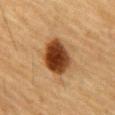Findings:
* follow-up — total-body-photography surveillance lesion; no biopsy
* lesion size — ≈5 mm
* illumination — cross-polarized
* image — ~15 mm tile from a whole-body skin photo
* body site — the abdomen
* patient — male, aged 83 or older